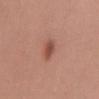Case summary:
- follow-up — total-body-photography surveillance lesion; no biopsy
- site — the mid back
- lighting — white-light
- automated lesion analysis — border irregularity of about 2 on a 0–10 scale, a color-variation rating of about 3.5/10, and a peripheral color-asymmetry measure near 1; a nevus-likeness score of about 95/100 and lesion-presence confidence of about 100/100
- patient — female, aged 43 to 47
- size — about 3 mm
- image source — 15 mm crop, total-body photography